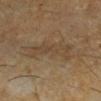| key | value |
|---|---|
| biopsy status | no biopsy performed (imaged during a skin exam) |
| subject | male, about 60 years old |
| TBP lesion metrics | an average lesion color of about L≈40 a*≈12 b*≈28 (CIELAB), a lesion–skin lightness drop of about 6, and a lesion-to-skin contrast of about 5 (normalized; higher = more distinct); a classifier nevus-likeness of about 0/100 and lesion-presence confidence of about 60/100 |
| anatomic site | the right lower leg |
| image source | ~15 mm crop, total-body skin-cancer survey |
| size | about 7 mm |
| illumination | cross-polarized |